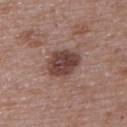No biopsy was performed on this lesion — it was imaged during a full skin examination and was not determined to be concerning.
A female patient approximately 65 years of age.
The total-body-photography lesion software estimated a lesion color around L≈42 a*≈19 b*≈22 in CIELAB, about 13 CIELAB-L* units darker than the surrounding skin, and a lesion-to-skin contrast of about 10 (normalized; higher = more distinct). The analysis additionally found border irregularity of about 1.5 on a 0–10 scale, a within-lesion color-variation index near 4/10, and a peripheral color-asymmetry measure near 1.5. And it measured a classifier nevus-likeness of about 45/100 and a detector confidence of about 100 out of 100 that the crop contains a lesion.
A 15 mm crop from a total-body photograph taken for skin-cancer surveillance.
Captured under white-light illumination.
On the upper back.